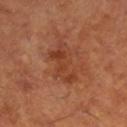| field | value |
|---|---|
| notes | catalogued during a skin exam; not biopsied |
| imaging modality | 15 mm crop, total-body photography |
| body site | the right lower leg |
| patient | male, aged 63–67 |
| automated lesion analysis | two-axis asymmetry of about 0.35; an average lesion color of about L≈41 a*≈27 b*≈33 (CIELAB), about 8 CIELAB-L* units darker than the surrounding skin, and a lesion-to-skin contrast of about 6.5 (normalized; higher = more distinct); a border-irregularity index near 5.5/10 and a color-variation rating of about 4/10 |